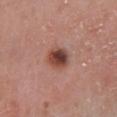Q: Was this lesion biopsied?
A: total-body-photography surveillance lesion; no biopsy
Q: What did automated image analysis measure?
A: an average lesion color of about L≈44 a*≈24 b*≈26 (CIELAB), roughly 15 lightness units darker than nearby skin, and a lesion-to-skin contrast of about 10.5 (normalized; higher = more distinct); an automated nevus-likeness rating near 100 out of 100 and lesion-presence confidence of about 100/100
Q: Lesion location?
A: the left lower leg
Q: Who is the patient?
A: male, about 80 years old
Q: What is the lesion's diameter?
A: ~3 mm (longest diameter)
Q: How was this image acquired?
A: ~15 mm tile from a whole-body skin photo